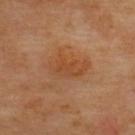workup = total-body-photography surveillance lesion; no biopsy
location = the upper back
patient = aged 63 to 67
lesion diameter = ≈4.5 mm
image-analysis metrics = a border-irregularity index near 4/10, internal color variation of about 2.5 on a 0–10 scale, and peripheral color asymmetry of about 1; a classifier nevus-likeness of about 10/100 and a detector confidence of about 100 out of 100 that the crop contains a lesion
imaging modality = 15 mm crop, total-body photography